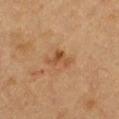Context:
The lesion's longest dimension is about 3.5 mm. The lesion is on the front of the torso. Cropped from a total-body skin-imaging series; the visible field is about 15 mm. A female subject roughly 40 years of age.